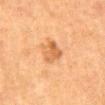Clinical impression:
Captured during whole-body skin photography for melanoma surveillance; the lesion was not biopsied.
Clinical summary:
Longest diameter approximately 3.5 mm. Cropped from a total-body skin-imaging series; the visible field is about 15 mm. The tile uses cross-polarized illumination. Located on the abdomen. The patient is a female roughly 50 years of age.